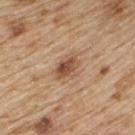{
  "biopsy_status": "not biopsied; imaged during a skin examination",
  "site": "upper back",
  "lesion_size": {
    "long_diameter_mm_approx": 3.5
  },
  "image": {
    "source": "total-body photography crop",
    "field_of_view_mm": 15
  },
  "patient": {
    "sex": "male",
    "age_approx": 70
  },
  "lighting": "white-light"
}